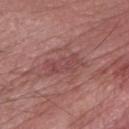No biopsy was performed on this lesion — it was imaged during a full skin examination and was not determined to be concerning.